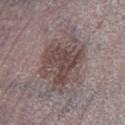Assessment: This lesion was catalogued during total-body skin photography and was not selected for biopsy. Context: Approximately 7 mm at its widest. A region of skin cropped from a whole-body photographic capture, roughly 15 mm wide. The lesion-visualizer software estimated an average lesion color of about L≈46 a*≈14 b*≈17 (CIELAB) and a lesion–skin lightness drop of about 10. And it measured a border-irregularity rating of about 4/10, a within-lesion color-variation index near 5.5/10, and radial color variation of about 2. The analysis additionally found a classifier nevus-likeness of about 25/100 and a lesion-detection confidence of about 60/100. On the left lower leg. A male patient aged 73–77. Captured under white-light illumination.